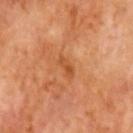Imaged with cross-polarized lighting. A 15 mm crop from a total-body photograph taken for skin-cancer surveillance. From the head or neck. A male patient, approximately 60 years of age.